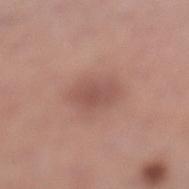Assessment:
Imaged during a routine full-body skin examination; the lesion was not biopsied and no histopathology is available.
Context:
An algorithmic analysis of the crop reported a lesion area of about 8 mm², an eccentricity of roughly 0.75, and a shape-asymmetry score of about 0.2 (0 = symmetric). It also reported border irregularity of about 2 on a 0–10 scale, a color-variation rating of about 2/10, and radial color variation of about 0.5. The analysis additionally found an automated nevus-likeness rating near 55 out of 100 and lesion-presence confidence of about 100/100. The lesion's longest dimension is about 4 mm. The patient is a female aged around 60. From the left lower leg. This image is a 15 mm lesion crop taken from a total-body photograph. The tile uses white-light illumination.